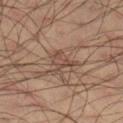– imaging modality: ~15 mm tile from a whole-body skin photo
– body site: the right lower leg
– subject: male, aged 33 to 37
– lighting: cross-polarized illumination
– automated metrics: a lesion area of about 5.5 mm², a shape eccentricity near 0.45, and a shape-asymmetry score of about 0.5 (0 = symmetric); an automated nevus-likeness rating near 0 out of 100 and lesion-presence confidence of about 65/100